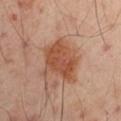Findings:
* workup · catalogued during a skin exam; not biopsied
* site · the arm
* subject · male, aged around 50
* image · ~15 mm crop, total-body skin-cancer survey